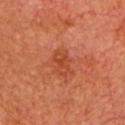| key | value |
|---|---|
| follow-up | imaged on a skin check; not biopsied |
| location | the head or neck |
| imaging modality | 15 mm crop, total-body photography |
| patient | male, about 85 years old or older |
| lighting | cross-polarized |
| TBP lesion metrics | a footprint of about 5.5 mm² and a symmetry-axis asymmetry near 0.25; border irregularity of about 3 on a 0–10 scale, a color-variation rating of about 2.5/10, and peripheral color asymmetry of about 0.5 |